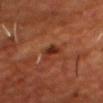No biopsy was performed on this lesion — it was imaged during a full skin examination and was not determined to be concerning. Located on the head or neck. A male patient, in their mid- to late 50s. A 15 mm crop from a total-body photograph taken for skin-cancer surveillance.